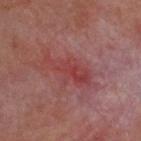Notes:
• image · 15 mm crop, total-body photography
• patient · male, aged 58–62
• illumination · cross-polarized
• location · the head or neck